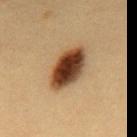Impression: Imaged during a routine full-body skin examination; the lesion was not biopsied and no histopathology is available. Background: Automated tile analysis of the lesion measured an area of roughly 14 mm², a shape eccentricity near 0.85, and a symmetry-axis asymmetry near 0.1. The software also gave a mean CIELAB color near L≈38 a*≈19 b*≈31, about 21 CIELAB-L* units darker than the surrounding skin, and a normalized lesion–skin contrast near 16. The software also gave border irregularity of about 1.5 on a 0–10 scale and a peripheral color-asymmetry measure near 2. The software also gave an automated nevus-likeness rating near 100 out of 100. A roughly 15 mm field-of-view crop from a total-body skin photograph. Captured under cross-polarized illumination. The subject is a female about 30 years old. The lesion is located on the upper back.